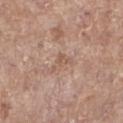Captured during whole-body skin photography for melanoma surveillance; the lesion was not biopsied. An algorithmic analysis of the crop reported an outline eccentricity of about 0.85 (0 = round, 1 = elongated) and two-axis asymmetry of about 0.6. Measured at roughly 3 mm in maximum diameter. A female subject, aged 73–77. A roughly 15 mm field-of-view crop from a total-body skin photograph. This is a white-light tile. On the left lower leg.